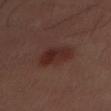Impression: No biopsy was performed on this lesion — it was imaged during a full skin examination and was not determined to be concerning. Context: The tile uses cross-polarized illumination. An algorithmic analysis of the crop reported an eccentricity of roughly 0.75 and a shape-asymmetry score of about 0.2 (0 = symmetric). And it measured a lesion color around L≈26 a*≈20 b*≈21 in CIELAB, roughly 7 lightness units darker than nearby skin, and a normalized lesion–skin contrast near 8. And it measured a border-irregularity index near 2.5/10, internal color variation of about 3.5 on a 0–10 scale, and a peripheral color-asymmetry measure near 1.5. Cropped from a whole-body photographic skin survey; the tile spans about 15 mm. From the abdomen. A male patient, aged approximately 40.